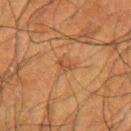<case>
  <biopsy_status>not biopsied; imaged during a skin examination</biopsy_status>
  <patient>
    <sex>male</sex>
    <age_approx>60</age_approx>
  </patient>
  <lesion_size>
    <long_diameter_mm_approx>2.5</long_diameter_mm_approx>
  </lesion_size>
  <lighting>cross-polarized</lighting>
  <site>right upper arm</site>
  <image>
    <source>total-body photography crop</source>
    <field_of_view_mm>15</field_of_view_mm>
  </image>
</case>